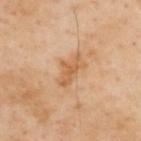Clinical impression:
The lesion was photographed on a routine skin check and not biopsied; there is no pathology result.
Acquisition and patient details:
A male subject aged 53–57. The lesion's longest dimension is about 4 mm. Imaged with cross-polarized lighting. From the upper back. Automated tile analysis of the lesion measured an average lesion color of about L≈61 a*≈21 b*≈38 (CIELAB), about 9 CIELAB-L* units darker than the surrounding skin, and a lesion-to-skin contrast of about 6.5 (normalized; higher = more distinct). The software also gave a border-irregularity index near 4.5/10 and peripheral color asymmetry of about 0.5. The analysis additionally found a nevus-likeness score of about 0/100 and a detector confidence of about 100 out of 100 that the crop contains a lesion. A roughly 15 mm field-of-view crop from a total-body skin photograph.